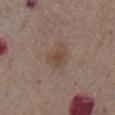<lesion>
<biopsy_status>not biopsied; imaged during a skin examination</biopsy_status>
<image>
  <source>total-body photography crop</source>
  <field_of_view_mm>15</field_of_view_mm>
</image>
<lesion_size>
  <long_diameter_mm_approx>3.0</long_diameter_mm_approx>
</lesion_size>
<site>chest</site>
<patient>
  <sex>male</sex>
  <age_approx>75</age_approx>
</patient>
<lighting>white-light</lighting>
<automated_metrics>
  <area_mm2_approx>5.5</area_mm2_approx>
  <eccentricity>0.7</eccentricity>
  <shape_asymmetry>0.35</shape_asymmetry>
</automated_metrics>
</lesion>